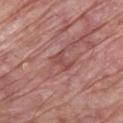Acquisition and patient details: This image is a 15 mm lesion crop taken from a total-body photograph. A male subject, aged 63–67. The lesion is on the chest. Captured under white-light illumination.